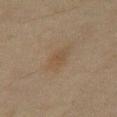Recorded during total-body skin imaging; not selected for excision or biopsy. Approximately 3.5 mm at its widest. Imaged with cross-polarized lighting. The total-body-photography lesion software estimated a lesion area of about 5 mm², an outline eccentricity of about 0.8 (0 = round, 1 = elongated), and two-axis asymmetry of about 0.3. It also reported an average lesion color of about L≈39 a*≈11 b*≈25 (CIELAB), a lesion–skin lightness drop of about 5, and a normalized border contrast of about 5. And it measured a border-irregularity index near 3/10, a color-variation rating of about 1.5/10, and radial color variation of about 0.5. The analysis additionally found a classifier nevus-likeness of about 15/100 and a detector confidence of about 100 out of 100 that the crop contains a lesion. The subject is a male aged 43 to 47. A region of skin cropped from a whole-body photographic capture, roughly 15 mm wide. From the abdomen.